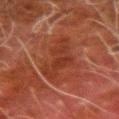The patient is a male about 80 years old. Automated tile analysis of the lesion measured a footprint of about 12 mm², a shape eccentricity near 0.95, and two-axis asymmetry of about 0.4. The software also gave a border-irregularity rating of about 6/10 and peripheral color asymmetry of about 0.5. A roughly 15 mm field-of-view crop from a total-body skin photograph. The lesion's longest dimension is about 7 mm. Imaged with cross-polarized lighting. The lesion is located on the right forearm.